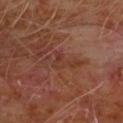workup: no biopsy performed (imaged during a skin exam)
subject: male, aged around 60
location: the chest
image source: 15 mm crop, total-body photography